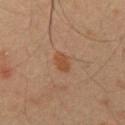The lesion was photographed on a routine skin check and not biopsied; there is no pathology result.
A male patient, approximately 50 years of age.
Approximately 2.5 mm at its widest.
The lesion-visualizer software estimated an eccentricity of roughly 0.8. It also reported a mean CIELAB color near L≈35 a*≈17 b*≈27, about 6 CIELAB-L* units darker than the surrounding skin, and a normalized border contrast of about 7.
The tile uses cross-polarized illumination.
From the chest.
Cropped from a whole-body photographic skin survey; the tile spans about 15 mm.